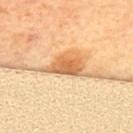The lesion was photographed on a routine skin check and not biopsied; there is no pathology result.
Approximately 4 mm at its widest.
The patient is a female roughly 65 years of age.
A 15 mm crop from a total-body photograph taken for skin-cancer surveillance.
On the upper back.